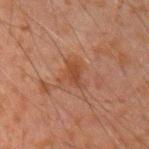Clinical summary:
Cropped from a total-body skin-imaging series; the visible field is about 15 mm. From the left upper arm. The lesion-visualizer software estimated about 6 CIELAB-L* units darker than the surrounding skin and a lesion-to-skin contrast of about 6 (normalized; higher = more distinct). The software also gave a classifier nevus-likeness of about 0/100 and lesion-presence confidence of about 100/100. This is a cross-polarized tile. A male subject, approximately 35 years of age.